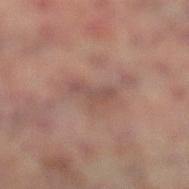Clinical impression: Recorded during total-body skin imaging; not selected for excision or biopsy.